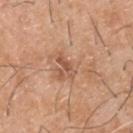A roughly 15 mm field-of-view crop from a total-body skin photograph. The lesion is located on the upper back. This is a white-light tile. Approximately 2.5 mm at its widest. A male subject, roughly 40 years of age.